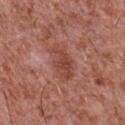biopsy status: catalogued during a skin exam; not biopsied.